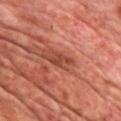This lesion was catalogued during total-body skin photography and was not selected for biopsy. Located on the chest. This is a cross-polarized tile. This image is a 15 mm lesion crop taken from a total-body photograph. The subject is a male aged 63–67.